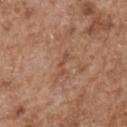{
  "biopsy_status": "not biopsied; imaged during a skin examination",
  "lighting": "white-light",
  "image": {
    "source": "total-body photography crop",
    "field_of_view_mm": 15
  },
  "patient": {
    "sex": "male",
    "age_approx": 65
  },
  "lesion_size": {
    "long_diameter_mm_approx": 3.0
  },
  "site": "upper back"
}